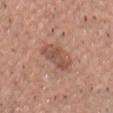Impression: Recorded during total-body skin imaging; not selected for excision or biopsy. Acquisition and patient details: Approximately 4 mm at its widest. A 15 mm crop from a total-body photograph taken for skin-cancer surveillance. Imaged with white-light lighting. The lesion is located on the chest. The patient is a male roughly 55 years of age.